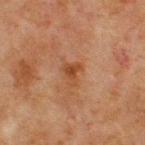workup=total-body-photography surveillance lesion; no biopsy.